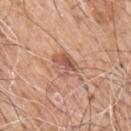workup: total-body-photography surveillance lesion; no biopsy